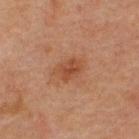Assessment:
Recorded during total-body skin imaging; not selected for excision or biopsy.
Clinical summary:
Automated tile analysis of the lesion measured a lesion area of about 6 mm² and a symmetry-axis asymmetry near 0.25. The software also gave an average lesion color of about L≈39 a*≈21 b*≈29 (CIELAB), about 7 CIELAB-L* units darker than the surrounding skin, and a normalized lesion–skin contrast near 6.5. It also reported a border-irregularity rating of about 2.5/10 and radial color variation of about 1. It also reported an automated nevus-likeness rating near 55 out of 100. This is a cross-polarized tile. This image is a 15 mm lesion crop taken from a total-body photograph. On the upper back. A male subject approximately 60 years of age.